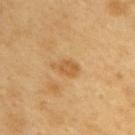image source: 15 mm crop, total-body photography; patient: male, in their 60s; location: the right upper arm; TBP lesion metrics: a border-irregularity rating of about 3/10.